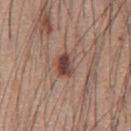Findings:
* follow-up: no biopsy performed (imaged during a skin exam)
* image: total-body-photography crop, ~15 mm field of view
* patient: male, approximately 60 years of age
* location: the chest
* tile lighting: white-light illumination